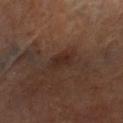Clinical impression: The lesion was photographed on a routine skin check and not biopsied; there is no pathology result. Clinical summary: A 15 mm close-up tile from a total-body photography series done for melanoma screening. A male patient in their mid-80s or older. An algorithmic analysis of the crop reported a border-irregularity index near 2.5/10, a color-variation rating of about 2.5/10, and a peripheral color-asymmetry measure near 1. And it measured an automated nevus-likeness rating near 10 out of 100 and lesion-presence confidence of about 100/100. Longest diameter approximately 3 mm. From the right lower leg. This is a cross-polarized tile.